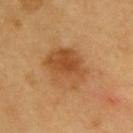Assessment:
Imaged during a routine full-body skin examination; the lesion was not biopsied and no histopathology is available.
Acquisition and patient details:
Cropped from a whole-body photographic skin survey; the tile spans about 15 mm. A male patient, aged 58–62. On the upper back. Longest diameter approximately 5.5 mm.